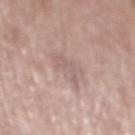workup — imaged on a skin check; not biopsied | automated lesion analysis — about 7 CIELAB-L* units darker than the surrounding skin and a lesion-to-skin contrast of about 4.5 (normalized; higher = more distinct) | patient — male, aged approximately 65 | acquisition — total-body-photography crop, ~15 mm field of view | tile lighting — white-light | anatomic site — the mid back.